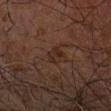Case summary:
• follow-up — imaged on a skin check; not biopsied
• subject — male, aged around 70
• lighting — cross-polarized illumination
• image-analysis metrics — a shape eccentricity near 0.7 and a shape-asymmetry score of about 0.25 (0 = symmetric); a mean CIELAB color near L≈21 a*≈14 b*≈19 and a normalized lesion–skin contrast near 6
• acquisition — ~15 mm crop, total-body skin-cancer survey
• size — ~2.5 mm (longest diameter)
• body site — the left forearm